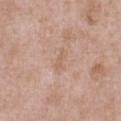Recorded during total-body skin imaging; not selected for excision or biopsy.
Captured under white-light illumination.
Located on the front of the torso.
A close-up tile cropped from a whole-body skin photograph, about 15 mm across.
The lesion-visualizer software estimated a shape eccentricity near 0.95 and a shape-asymmetry score of about 0.45 (0 = symmetric).
A male patient, approximately 50 years of age.